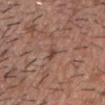Clinical impression: Imaged during a routine full-body skin examination; the lesion was not biopsied and no histopathology is available. Image and clinical context: A close-up tile cropped from a whole-body skin photograph, about 15 mm across. This is a white-light tile. From the chest. A male patient, approximately 50 years of age.